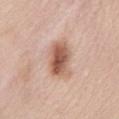Clinical impression: Part of a total-body skin-imaging series; this lesion was reviewed on a skin check and was not flagged for biopsy. Acquisition and patient details: Automated image analysis of the tile measured an area of roughly 11 mm² and a shape eccentricity near 0.8. And it measured a border-irregularity rating of about 2.5/10, a within-lesion color-variation index near 6/10, and radial color variation of about 2. It also reported a detector confidence of about 100 out of 100 that the crop contains a lesion. The lesion's longest dimension is about 4.5 mm. A female patient about 50 years old. Cropped from a total-body skin-imaging series; the visible field is about 15 mm. From the back.